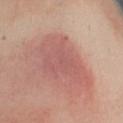Clinical impression: The lesion was tiled from a total-body skin photograph and was not biopsied. Background: The patient is a female about 40 years old. The lesion is located on the left upper arm. A lesion tile, about 15 mm wide, cut from a 3D total-body photograph. The lesion-visualizer software estimated an average lesion color of about L≈58 a*≈25 b*≈25 (CIELAB) and roughly 9 lightness units darker than nearby skin. The software also gave border irregularity of about 5 on a 0–10 scale, a within-lesion color-variation index near 2.5/10, and peripheral color asymmetry of about 1. And it measured a classifier nevus-likeness of about 15/100 and lesion-presence confidence of about 100/100.